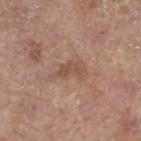notes = total-body-photography surveillance lesion; no biopsy | acquisition = ~15 mm crop, total-body skin-cancer survey | location = the left lower leg | subject = female, approximately 75 years of age.